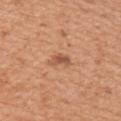| key | value |
|---|---|
| notes | no biopsy performed (imaged during a skin exam) |
| patient | male, in their mid-50s |
| size | ~2.5 mm (longest diameter) |
| tile lighting | white-light |
| anatomic site | the right upper arm |
| acquisition | 15 mm crop, total-body photography |